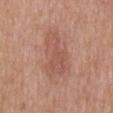No biopsy was performed on this lesion — it was imaged during a full skin examination and was not determined to be concerning. The lesion is located on the mid back. Automated tile analysis of the lesion measured a lesion area of about 14 mm², an outline eccentricity of about 0.85 (0 = round, 1 = elongated), and a shape-asymmetry score of about 0.45 (0 = symmetric). It also reported an automated nevus-likeness rating near 5 out of 100. A male patient, aged 53 to 57. Longest diameter approximately 6 mm. This image is a 15 mm lesion crop taken from a total-body photograph.